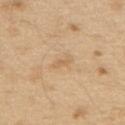Assessment: The lesion was tiled from a total-body skin photograph and was not biopsied. Context: Located on the upper back. The subject is a male roughly 70 years of age. An algorithmic analysis of the crop reported an eccentricity of roughly 0.95 and a symmetry-axis asymmetry near 0.25. The software also gave a mean CIELAB color near L≈64 a*≈16 b*≈38 and a normalized lesion–skin contrast near 5. And it measured a detector confidence of about 100 out of 100 that the crop contains a lesion. The tile uses white-light illumination. Cropped from a whole-body photographic skin survey; the tile spans about 15 mm.